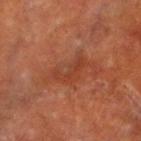Captured during whole-body skin photography for melanoma surveillance; the lesion was not biopsied. Located on the right lower leg. A 15 mm close-up tile from a total-body photography series done for melanoma screening. The subject is a male in their 70s. Approximately 4 mm at its widest.